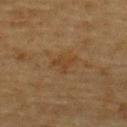Case summary:
- follow-up · imaged on a skin check; not biopsied
- image-analysis metrics · an area of roughly 3.5 mm², an eccentricity of roughly 0.7, and two-axis asymmetry of about 0.4; a mean CIELAB color near L≈35 a*≈16 b*≈31, about 5 CIELAB-L* units darker than the surrounding skin, and a normalized lesion–skin contrast near 5.5; an automated nevus-likeness rating near 0 out of 100 and a detector confidence of about 100 out of 100 that the crop contains a lesion
- image · ~15 mm crop, total-body skin-cancer survey
- lesion size · ~2.5 mm (longest diameter)
- illumination · cross-polarized
- subject · male, approximately 85 years of age or older
- site · the upper back